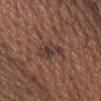Assessment:
Imaged during a routine full-body skin examination; the lesion was not biopsied and no histopathology is available.
Context:
The recorded lesion diameter is about 4 mm. A male subject, aged approximately 65. The lesion is located on the chest. A roughly 15 mm field-of-view crop from a total-body skin photograph. This is a white-light tile.